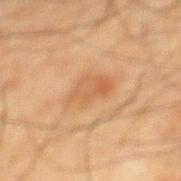Notes:
– notes · catalogued during a skin exam; not biopsied
– size · about 5 mm
– acquisition · ~15 mm tile from a whole-body skin photo
– subject · male, aged 63–67
– body site · the mid back